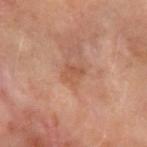Case summary:
• workup · total-body-photography surveillance lesion; no biopsy
• subject · female, aged 58 to 62
• image source · total-body-photography crop, ~15 mm field of view
• site · the left forearm
• image-analysis metrics · an area of roughly 4.5 mm², an eccentricity of roughly 0.6, and a symmetry-axis asymmetry near 0.3; a border-irregularity index near 3/10 and a color-variation rating of about 2/10